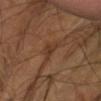biopsy_status: not biopsied; imaged during a skin examination
site: arm
image:
  source: total-body photography crop
  field_of_view_mm: 15
patient:
  sex: male
  age_approx: 60